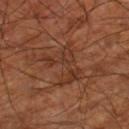Recorded during total-body skin imaging; not selected for excision or biopsy. The lesion is on the leg. A patient aged 63–67. A 15 mm crop from a total-body photograph taken for skin-cancer surveillance. This is a cross-polarized tile.